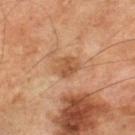{"biopsy_status": "not biopsied; imaged during a skin examination", "image": {"source": "total-body photography crop", "field_of_view_mm": 15}, "lesion_size": {"long_diameter_mm_approx": 2.5}, "patient": {"sex": "male", "age_approx": 65}, "lighting": "cross-polarized", "site": "right lower leg"}